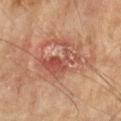This lesion was catalogued during total-body skin photography and was not selected for biopsy. Automated tile analysis of the lesion measured a lesion area of about 9.5 mm² and two-axis asymmetry of about 0.45. The analysis additionally found an average lesion color of about L≈42 a*≈23 b*≈25 (CIELAB), roughly 8 lightness units darker than nearby skin, and a lesion-to-skin contrast of about 6.5 (normalized; higher = more distinct). The software also gave a border-irregularity index near 8/10, a within-lesion color-variation index near 4/10, and peripheral color asymmetry of about 1.5. And it measured a nevus-likeness score of about 0/100. The patient is a male aged 73–77. A lesion tile, about 15 mm wide, cut from a 3D total-body photograph. The lesion is on the left upper arm.